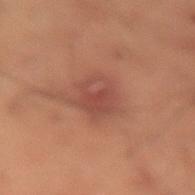biopsy_status: not biopsied; imaged during a skin examination
automated_metrics:
  vs_skin_darker_L: 6.0
  vs_skin_contrast_norm: 6.0
  nevus_likeness_0_100: 10
image:
  source: total-body photography crop
  field_of_view_mm: 15
patient:
  sex: male
  age_approx: 50
lesion_size:
  long_diameter_mm_approx: 4.0
site: lower back
lighting: cross-polarized